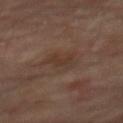Part of a total-body skin-imaging series; this lesion was reviewed on a skin check and was not flagged for biopsy. A 15 mm crop from a total-body photograph taken for skin-cancer surveillance. On the mid back. The tile uses cross-polarized illumination. The patient is a male aged 63 to 67.